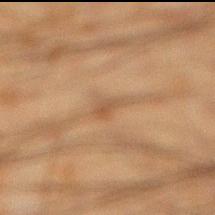| key | value |
|---|---|
| notes | imaged on a skin check; not biopsied |
| patient | male, in their mid-30s |
| image | 15 mm crop, total-body photography |
| location | the right lower leg |